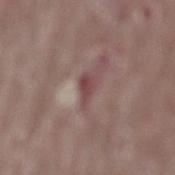Assessment:
The lesion was photographed on a routine skin check and not biopsied; there is no pathology result.
Clinical summary:
A 15 mm close-up extracted from a 3D total-body photography capture. Captured under white-light illumination. From the mid back. A male patient in their 40s. The total-body-photography lesion software estimated a border-irregularity rating of about 4/10 and radial color variation of about 1. It also reported lesion-presence confidence of about 65/100. Measured at roughly 2.5 mm in maximum diameter.